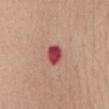Clinical impression:
No biopsy was performed on this lesion — it was imaged during a full skin examination and was not determined to be concerning.
Context:
A 15 mm crop from a total-body photograph taken for skin-cancer surveillance. A female subject, aged 58–62. Located on the abdomen.